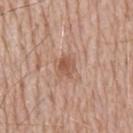Q: Is there a histopathology result?
A: no biopsy performed (imaged during a skin exam)
Q: Where on the body is the lesion?
A: the mid back
Q: Automated lesion metrics?
A: a lesion area of about 4 mm², an eccentricity of roughly 0.65, and two-axis asymmetry of about 0.2; a border-irregularity index near 2/10, a color-variation rating of about 2.5/10, and peripheral color asymmetry of about 1
Q: How was this image acquired?
A: ~15 mm tile from a whole-body skin photo
Q: What lighting was used for the tile?
A: white-light
Q: What are the patient's age and sex?
A: male, aged 78 to 82
Q: How large is the lesion?
A: about 2.5 mm